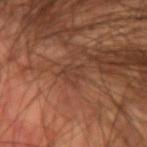notes=catalogued during a skin exam; not biopsied
patient=male, aged 58 to 62
diameter=≈2.5 mm
imaging modality=15 mm crop, total-body photography
illumination=cross-polarized illumination
site=the left upper arm